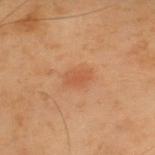Findings:
* notes · imaged on a skin check; not biopsied
* subject · male, in their mid-50s
* body site · the upper back
* illumination · cross-polarized illumination
* acquisition · total-body-photography crop, ~15 mm field of view
* lesion size · ≈3 mm